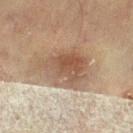No biopsy was performed on this lesion — it was imaged during a full skin examination and was not determined to be concerning. The total-body-photography lesion software estimated a shape eccentricity near 0.7 and a shape-asymmetry score of about 0.3 (0 = symmetric). And it measured internal color variation of about 5 on a 0–10 scale and radial color variation of about 1.5. A 15 mm close-up tile from a total-body photography series done for melanoma screening. Captured under cross-polarized illumination. The patient is a male aged 68–72. The lesion is on the front of the torso. About 6 mm across.